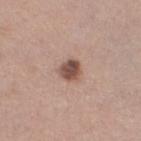Assessment:
Captured during whole-body skin photography for melanoma surveillance; the lesion was not biopsied.
Background:
From the leg. A 15 mm close-up tile from a total-body photography series done for melanoma screening. Captured under white-light illumination. A female subject, aged 53 to 57.